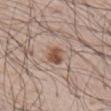Q: Was this lesion biopsied?
A: imaged on a skin check; not biopsied
Q: How was the tile lit?
A: white-light
Q: Where on the body is the lesion?
A: the upper back
Q: How large is the lesion?
A: ~3 mm (longest diameter)
Q: Who is the patient?
A: male, in their mid- to late 60s
Q: What is the imaging modality?
A: ~15 mm crop, total-body skin-cancer survey